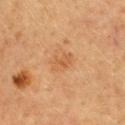Part of a total-body skin-imaging series; this lesion was reviewed on a skin check and was not flagged for biopsy. A female patient roughly 60 years of age. Longest diameter approximately 3 mm. This is a cross-polarized tile. A 15 mm crop from a total-body photograph taken for skin-cancer surveillance. From the back.